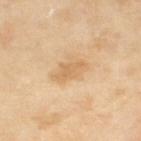Imaged during a routine full-body skin examination; the lesion was not biopsied and no histopathology is available. The lesion is located on the leg. An algorithmic analysis of the crop reported an outline eccentricity of about 0.8 (0 = round, 1 = elongated) and two-axis asymmetry of about 0.3. And it measured an automated nevus-likeness rating near 0 out of 100 and lesion-presence confidence of about 100/100. A region of skin cropped from a whole-body photographic capture, roughly 15 mm wide. Imaged with cross-polarized lighting. The patient is a female about 70 years old. The lesion's longest dimension is about 3.5 mm.